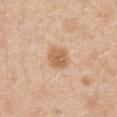Q: Was a biopsy performed?
A: imaged on a skin check; not biopsied
Q: How large is the lesion?
A: ~2.5 mm (longest diameter)
Q: What is the imaging modality?
A: total-body-photography crop, ~15 mm field of view
Q: Where on the body is the lesion?
A: the chest
Q: Who is the patient?
A: female, aged around 40
Q: Illumination type?
A: white-light illumination